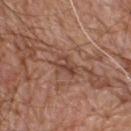This lesion was catalogued during total-body skin photography and was not selected for biopsy. Automated image analysis of the tile measured an area of roughly 5.5 mm² and an outline eccentricity of about 0.75 (0 = round, 1 = elongated). The analysis additionally found an average lesion color of about L≈45 a*≈21 b*≈27 (CIELAB), about 8 CIELAB-L* units darker than the surrounding skin, and a normalized lesion–skin contrast near 6.5. And it measured border irregularity of about 3.5 on a 0–10 scale, a within-lesion color-variation index near 5/10, and a peripheral color-asymmetry measure near 2. About 3 mm across. This image is a 15 mm lesion crop taken from a total-body photograph. From the back. A male patient aged approximately 80. Imaged with white-light lighting.